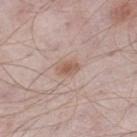biopsy status: catalogued during a skin exam; not biopsied
lesion size: ~2.5 mm (longest diameter)
patient: male, aged approximately 55
illumination: white-light
site: the left thigh
image source: 15 mm crop, total-body photography